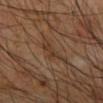Captured during whole-body skin photography for melanoma surveillance; the lesion was not biopsied. The subject is a male aged 68–72. A 15 mm close-up tile from a total-body photography series done for melanoma screening. Located on the right forearm. The tile uses cross-polarized illumination. An algorithmic analysis of the crop reported an area of roughly 3 mm², an eccentricity of roughly 0.85, and a symmetry-axis asymmetry near 0.5. The software also gave a border-irregularity index near 5.5/10, a within-lesion color-variation index near 0/10, and radial color variation of about 0. Measured at roughly 3 mm in maximum diameter.